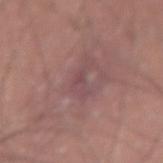Clinical impression:
Recorded during total-body skin imaging; not selected for excision or biopsy.
Acquisition and patient details:
A male patient, aged around 25. A 15 mm crop from a total-body photograph taken for skin-cancer surveillance. Measured at roughly 3.5 mm in maximum diameter. The lesion-visualizer software estimated a lesion area of about 4 mm², an outline eccentricity of about 0.85 (0 = round, 1 = elongated), and a symmetry-axis asymmetry near 0.55. It also reported a border-irregularity index near 6.5/10, a within-lesion color-variation index near 2/10, and a peripheral color-asymmetry measure near 0.5. Located on the lower back. This is a white-light tile.